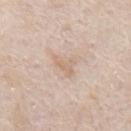Imaged during a routine full-body skin examination; the lesion was not biopsied and no histopathology is available. Captured under white-light illumination. From the mid back. A male patient, in their mid- to late 60s. The total-body-photography lesion software estimated a symmetry-axis asymmetry near 0.55. The analysis additionally found a mean CIELAB color near L≈67 a*≈15 b*≈28, about 7 CIELAB-L* units darker than the surrounding skin, and a normalized border contrast of about 5. The software also gave a classifier nevus-likeness of about 0/100 and lesion-presence confidence of about 95/100. Cropped from a total-body skin-imaging series; the visible field is about 15 mm.